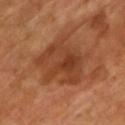<case>
<biopsy_status>not biopsied; imaged during a skin examination</biopsy_status>
<lesion_size>
  <long_diameter_mm_approx>6.5</long_diameter_mm_approx>
</lesion_size>
<site>chest</site>
<patient>
  <sex>male</sex>
  <age_approx>65</age_approx>
</patient>
<automated_metrics>
  <border_irregularity_0_10>5.0</border_irregularity_0_10>
  <color_variation_0_10>5.5</color_variation_0_10>
  <nevus_likeness_0_100>10</nevus_likeness_0_100>
</automated_metrics>
<image>
  <source>total-body photography crop</source>
  <field_of_view_mm>15</field_of_view_mm>
</image>
<lighting>cross-polarized</lighting>
</case>